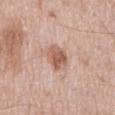Image and clinical context: The patient is a male aged around 60. Cropped from a whole-body photographic skin survey; the tile spans about 15 mm. About 3.5 mm across. An algorithmic analysis of the crop reported about 12 CIELAB-L* units darker than the surrounding skin and a lesion-to-skin contrast of about 8 (normalized; higher = more distinct). The analysis additionally found a nevus-likeness score of about 45/100 and a detector confidence of about 100 out of 100 that the crop contains a lesion. This is a white-light tile. The lesion is on the mid back.